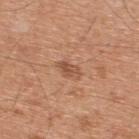Captured during whole-body skin photography for melanoma surveillance; the lesion was not biopsied. The lesion is on the upper back. A region of skin cropped from a whole-body photographic capture, roughly 15 mm wide. A male patient, roughly 45 years of age. Automated image analysis of the tile measured an area of roughly 3.5 mm², an eccentricity of roughly 0.75, and a symmetry-axis asymmetry near 0.35. It also reported a border-irregularity index near 3.5/10, a color-variation rating of about 2.5/10, and radial color variation of about 1. It also reported an automated nevus-likeness rating near 15 out of 100. The tile uses white-light illumination. Measured at roughly 2.5 mm in maximum diameter.